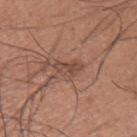workup: catalogued during a skin exam; not biopsied
patient: male, aged 33 to 37
imaging modality: 15 mm crop, total-body photography
TBP lesion metrics: an outline eccentricity of about 0.8 (0 = round, 1 = elongated) and a symmetry-axis asymmetry near 0.3; a mean CIELAB color near L≈47 a*≈20 b*≈26, about 8 CIELAB-L* units darker than the surrounding skin, and a normalized border contrast of about 6; a border-irregularity index near 3.5/10, a within-lesion color-variation index near 3/10, and a peripheral color-asymmetry measure near 1
tile lighting: white-light
diameter: ~3 mm (longest diameter)
location: the left upper arm